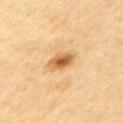| field | value |
|---|---|
| subject | male, aged 58 to 62 |
| acquisition | ~15 mm tile from a whole-body skin photo |
| automated lesion analysis | a shape eccentricity near 0.8 and two-axis asymmetry of about 0.2; border irregularity of about 2 on a 0–10 scale, a within-lesion color-variation index near 4.5/10, and peripheral color asymmetry of about 1 |
| lesion diameter | about 3.5 mm |